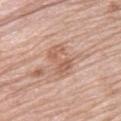  biopsy_status: not biopsied; imaged during a skin examination
  lesion_size:
    long_diameter_mm_approx: 4.0
  patient:
    sex: female
    age_approx: 65
  lighting: white-light
  image:
    source: total-body photography crop
    field_of_view_mm: 15
  automated_metrics:
    cielab_L: 60
    cielab_a: 21
    cielab_b: 30
    vs_skin_darker_L: 9.0
    vs_skin_contrast_norm: 6.0
    border_irregularity_0_10: 6.0
    color_variation_0_10: 2.5
    peripheral_color_asymmetry: 1.0
    lesion_detection_confidence_0_100: 100
  site: upper back